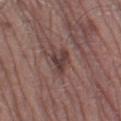Case summary:
- follow-up: catalogued during a skin exam; not biopsied
- lesion size: ≈3.5 mm
- imaging modality: total-body-photography crop, ~15 mm field of view
- site: the left thigh
- subject: male, approximately 65 years of age